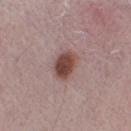<record>
<lighting>white-light</lighting>
<site>arm</site>
<image>
  <source>total-body photography crop</source>
  <field_of_view_mm>15</field_of_view_mm>
</image>
<patient>
  <sex>male</sex>
  <age_approx>70</age_approx>
</patient>
</record>